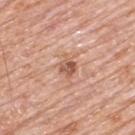workup = imaged on a skin check; not biopsied | patient = male, in their 80s | acquisition = 15 mm crop, total-body photography | location = the upper back.